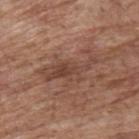<case>
<biopsy_status>not biopsied; imaged during a skin examination</biopsy_status>
<lighting>white-light</lighting>
<automated_metrics>
  <area_mm2_approx>13.0</area_mm2_approx>
  <eccentricity>0.9</eccentricity>
  <shape_asymmetry>0.5</shape_asymmetry>
  <vs_skin_contrast_norm>7.0</vs_skin_contrast_norm>
  <border_irregularity_0_10>9.0</border_irregularity_0_10>
  <color_variation_0_10>4.0</color_variation_0_10>
  <peripheral_color_asymmetry>1.5</peripheral_color_asymmetry>
  <nevus_likeness_0_100>0</nevus_likeness_0_100>
  <lesion_detection_confidence_0_100>90</lesion_detection_confidence_0_100>
</automated_metrics>
<patient>
  <sex>male</sex>
  <age_approx>70</age_approx>
</patient>
<site>upper back</site>
<lesion_size>
  <long_diameter_mm_approx>7.0</long_diameter_mm_approx>
</lesion_size>
<image>
  <source>total-body photography crop</source>
  <field_of_view_mm>15</field_of_view_mm>
</image>
</case>